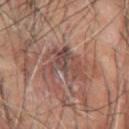biopsy_status: not biopsied; imaged during a skin examination
patient:
  sex: male
  age_approx: 65
site: left forearm
lesion_size:
  long_diameter_mm_approx: 5.5
image:
  source: total-body photography crop
  field_of_view_mm: 15